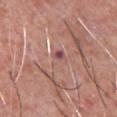• follow-up: total-body-photography surveillance lesion; no biopsy
• diameter: about 2.5 mm
• patient: male, aged approximately 55
• tile lighting: white-light illumination
• location: the chest
• imaging modality: ~15 mm tile from a whole-body skin photo